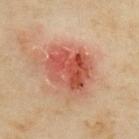notes: no biopsy performed (imaged during a skin exam)
size: about 5.5 mm
image-analysis metrics: a classifier nevus-likeness of about 5/100 and a lesion-detection confidence of about 100/100
image source: ~15 mm tile from a whole-body skin photo
site: the back
patient: female, aged around 35
lighting: cross-polarized